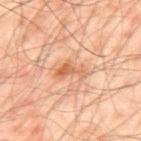biopsy_status: not biopsied; imaged during a skin examination
site: upper back
patient:
  sex: male
  age_approx: 50
image:
  source: total-body photography crop
  field_of_view_mm: 15
lighting: cross-polarized